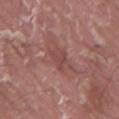follow-up: no biopsy performed (imaged during a skin exam)
image-analysis metrics: a lesion area of about 4 mm² and an eccentricity of roughly 0.5; a border-irregularity index near 4/10, internal color variation of about 1.5 on a 0–10 scale, and peripheral color asymmetry of about 0.5
image: total-body-photography crop, ~15 mm field of view
subject: male, aged approximately 40
site: the mid back
lesion size: about 2.5 mm